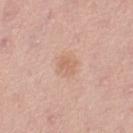{"biopsy_status": "not biopsied; imaged during a skin examination", "site": "leg", "lesion_size": {"long_diameter_mm_approx": 2.5}, "patient": {"sex": "female", "age_approx": 50}, "lighting": "white-light", "image": {"source": "total-body photography crop", "field_of_view_mm": 15}}